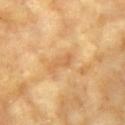Clinical impression:
Recorded during total-body skin imaging; not selected for excision or biopsy.
Clinical summary:
This is a cross-polarized tile. From the right upper arm. About 3.5 mm across. A female patient, aged 73–77. A region of skin cropped from a whole-body photographic capture, roughly 15 mm wide. Automated image analysis of the tile measured roughly 7 lightness units darker than nearby skin and a normalized border contrast of about 5. It also reported a classifier nevus-likeness of about 0/100 and a detector confidence of about 100 out of 100 that the crop contains a lesion.